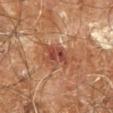Impression:
Part of a total-body skin-imaging series; this lesion was reviewed on a skin check and was not flagged for biopsy.
Image and clinical context:
A male patient aged 58–62. On the right leg. Cropped from a total-body skin-imaging series; the visible field is about 15 mm.